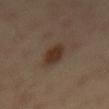Q: Was this lesion biopsied?
A: total-body-photography surveillance lesion; no biopsy
Q: What are the patient's age and sex?
A: male, in their mid- to late 40s
Q: Lesion location?
A: the mid back
Q: What is the imaging modality?
A: ~15 mm crop, total-body skin-cancer survey
Q: How was the tile lit?
A: cross-polarized illumination
Q: Lesion size?
A: about 4.5 mm